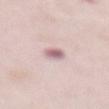No biopsy was performed on this lesion — it was imaged during a full skin examination and was not determined to be concerning. Located on the abdomen. A female patient aged 63 to 67. Measured at roughly 3 mm in maximum diameter. This is a white-light tile. A roughly 15 mm field-of-view crop from a total-body skin photograph.